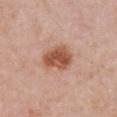Notes:
• biopsy status · catalogued during a skin exam; not biopsied
• anatomic site · the chest
• image source · ~15 mm crop, total-body skin-cancer survey
• subject · female, in their 50s
• lighting · white-light illumination